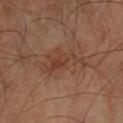Findings:
- follow-up: catalogued during a skin exam; not biopsied
- site: the left forearm
- illumination: cross-polarized
- automated metrics: border irregularity of about 9 on a 0–10 scale, internal color variation of about 2.5 on a 0–10 scale, and a peripheral color-asymmetry measure near 0.5
- lesion diameter: ~5.5 mm (longest diameter)
- imaging modality: 15 mm crop, total-body photography
- subject: male, approximately 70 years of age